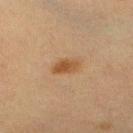Q: Was this lesion biopsied?
A: total-body-photography surveillance lesion; no biopsy
Q: Patient demographics?
A: female, roughly 55 years of age
Q: What is the imaging modality?
A: ~15 mm tile from a whole-body skin photo
Q: What is the lesion's diameter?
A: ≈3.5 mm
Q: What is the anatomic site?
A: the right lower leg
Q: Illumination type?
A: cross-polarized